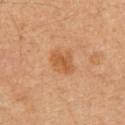Q: What is the imaging modality?
A: 15 mm crop, total-body photography
Q: How large is the lesion?
A: about 3 mm
Q: What lighting was used for the tile?
A: cross-polarized illumination
Q: Lesion location?
A: the mid back
Q: Patient demographics?
A: male, aged around 60